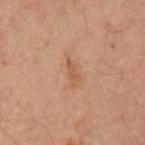| field | value |
|---|---|
| follow-up | imaged on a skin check; not biopsied |
| lesion size | ≈3 mm |
| imaging modality | ~15 mm tile from a whole-body skin photo |
| subject | male, approximately 50 years of age |
| location | the left upper arm |
| automated metrics | an area of roughly 3 mm², an eccentricity of roughly 0.95, and two-axis asymmetry of about 0.3; a mean CIELAB color near L≈49 a*≈20 b*≈31 and about 7 CIELAB-L* units darker than the surrounding skin; a classifier nevus-likeness of about 5/100 and a lesion-detection confidence of about 100/100 |
| illumination | cross-polarized illumination |